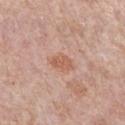patient: female, about 65 years old | site: the left forearm | imaging modality: ~15 mm tile from a whole-body skin photo.